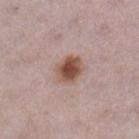Notes:
– workup — catalogued during a skin exam; not biopsied
– imaging modality — ~15 mm crop, total-body skin-cancer survey
– illumination — white-light illumination
– anatomic site — the leg
– size — ≈3.5 mm
– automated metrics — an eccentricity of roughly 0.65
– subject — female, aged around 30